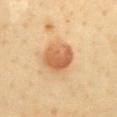notes — total-body-photography surveillance lesion; no biopsy
size — ~4 mm (longest diameter)
subject — female, aged 48–52
anatomic site — the chest
acquisition — ~15 mm crop, total-body skin-cancer survey
TBP lesion metrics — border irregularity of about 1 on a 0–10 scale, internal color variation of about 5 on a 0–10 scale, and radial color variation of about 2; a classifier nevus-likeness of about 100/100 and lesion-presence confidence of about 100/100